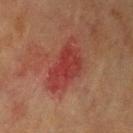Acquisition and patient details:
The patient is a female aged 78 to 82. Located on the left upper arm. The tile uses cross-polarized illumination. The total-body-photography lesion software estimated a footprint of about 16 mm² and an eccentricity of roughly 0.85. The software also gave a lesion color around L≈34 a*≈29 b*≈25 in CIELAB and a lesion-to-skin contrast of about 7 (normalized; higher = more distinct). The analysis additionally found a border-irregularity index near 4.5/10, a color-variation rating of about 3/10, and radial color variation of about 1. The software also gave a classifier nevus-likeness of about 0/100. This image is a 15 mm lesion crop taken from a total-body photograph. About 6.5 mm across.